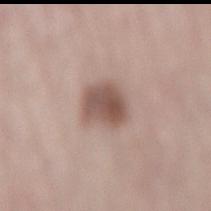On the right lower leg.
A female subject, approximately 50 years of age.
This image is a 15 mm lesion crop taken from a total-body photograph.